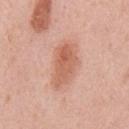Notes:
- size: about 6 mm
- TBP lesion metrics: a footprint of about 14 mm², an eccentricity of roughly 0.85, and a symmetry-axis asymmetry near 0.2; a mean CIELAB color near L≈62 a*≈24 b*≈31, a lesion–skin lightness drop of about 10, and a normalized border contrast of about 7; lesion-presence confidence of about 100/100
- tile lighting: white-light
- patient: male, in their mid-50s
- image source: total-body-photography crop, ~15 mm field of view
- anatomic site: the chest